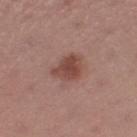follow-up = imaged on a skin check; not biopsied
subject = female, roughly 55 years of age
size = about 3.5 mm
acquisition = 15 mm crop, total-body photography
TBP lesion metrics = a border-irregularity rating of about 2.5/10 and radial color variation of about 1; a detector confidence of about 100 out of 100 that the crop contains a lesion
site = the left thigh